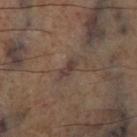Captured during whole-body skin photography for melanoma surveillance; the lesion was not biopsied.
Cropped from a total-body skin-imaging series; the visible field is about 15 mm.
Automated image analysis of the tile measured a lesion area of about 3 mm², an eccentricity of roughly 0.9, and a shape-asymmetry score of about 0.35 (0 = symmetric). The analysis additionally found an average lesion color of about L≈38 a*≈15 b*≈19 (CIELAB), a lesion–skin lightness drop of about 8, and a normalized border contrast of about 8.
On the right lower leg.
This is a cross-polarized tile.
The recorded lesion diameter is about 3 mm.